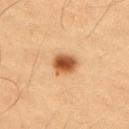{
  "biopsy_status": "not biopsied; imaged during a skin examination",
  "site": "right upper arm",
  "automated_metrics": {
    "vs_skin_darker_L": 17.0,
    "vs_skin_contrast_norm": 11.0,
    "color_variation_0_10": 5.5,
    "peripheral_color_asymmetry": 1.5,
    "nevus_likeness_0_100": 100,
    "lesion_detection_confidence_0_100": 100
  },
  "image": {
    "source": "total-body photography crop",
    "field_of_view_mm": 15
  },
  "patient": {
    "sex": "male",
    "age_approx": 55
  },
  "lighting": "cross-polarized"
}